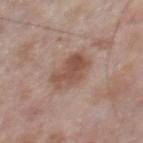notes: total-body-photography surveillance lesion; no biopsy | lighting: white-light | location: the chest | subject: male, aged 73–77 | imaging modality: total-body-photography crop, ~15 mm field of view | diameter: ≈4 mm.